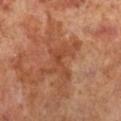Acquisition and patient details: A 15 mm crop from a total-body photograph taken for skin-cancer surveillance. The lesion is located on the right lower leg. A female subject aged 63–67. This is a cross-polarized tile. Approximately 6 mm at its widest.